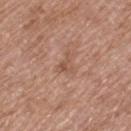{
  "biopsy_status": "not biopsied; imaged during a skin examination",
  "lesion_size": {
    "long_diameter_mm_approx": 2.5
  },
  "site": "left thigh",
  "automated_metrics": {
    "cielab_L": 53,
    "cielab_a": 21,
    "cielab_b": 29,
    "vs_skin_darker_L": 7.0,
    "vs_skin_contrast_norm": 5.5,
    "border_irregularity_0_10": 6.0,
    "color_variation_0_10": 1.0
  },
  "patient": {
    "sex": "female",
    "age_approx": 75
  },
  "image": {
    "source": "total-body photography crop",
    "field_of_view_mm": 15
  },
  "lighting": "white-light"
}